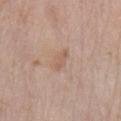{"biopsy_status": "not biopsied; imaged during a skin examination", "lesion_size": {"long_diameter_mm_approx": 3.0}, "lighting": "white-light", "automated_metrics": {"area_mm2_approx": 3.5, "eccentricity": 0.9, "cielab_L": 59, "cielab_a": 18, "cielab_b": 28, "vs_skin_darker_L": 6.0, "vs_skin_contrast_norm": 5.0, "border_irregularity_0_10": 3.5, "color_variation_0_10": 1.5, "peripheral_color_asymmetry": 0.5}, "patient": {"sex": "female", "age_approx": 70}, "site": "left lower leg", "image": {"source": "total-body photography crop", "field_of_view_mm": 15}}